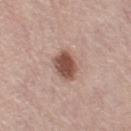The patient is a female aged around 65. Automated tile analysis of the lesion measured an area of roughly 8 mm², an eccentricity of roughly 0.8, and a shape-asymmetry score of about 0.2 (0 = symmetric). The analysis additionally found a lesion color around L≈51 a*≈21 b*≈26 in CIELAB and a lesion–skin lightness drop of about 15. The software also gave border irregularity of about 2 on a 0–10 scale, internal color variation of about 4 on a 0–10 scale, and radial color variation of about 1. The software also gave a nevus-likeness score of about 100/100 and a lesion-detection confidence of about 100/100. From the left thigh. A 15 mm close-up tile from a total-body photography series done for melanoma screening. The tile uses white-light illumination. Measured at roughly 4 mm in maximum diameter.